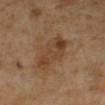A female subject, aged 48–52.
A 15 mm close-up tile from a total-body photography series done for melanoma screening.
On the right lower leg.